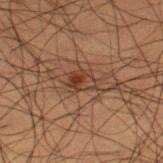This lesion was catalogued during total-body skin photography and was not selected for biopsy.
A male subject, roughly 50 years of age.
Longest diameter approximately 2.5 mm.
The tile uses cross-polarized illumination.
A roughly 15 mm field-of-view crop from a total-body skin photograph.
On the left thigh.